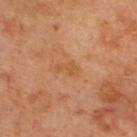This lesion was catalogued during total-body skin photography and was not selected for biopsy. Automated tile analysis of the lesion measured a mean CIELAB color near L≈53 a*≈23 b*≈39, about 6 CIELAB-L* units darker than the surrounding skin, and a normalized border contrast of about 5. Imaged with cross-polarized lighting. A region of skin cropped from a whole-body photographic capture, roughly 15 mm wide. The lesion is on the upper back. The patient is in their mid- to late 60s.